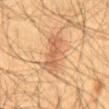| field | value |
|---|---|
| biopsy status | catalogued during a skin exam; not biopsied |
| image-analysis metrics | a lesion area of about 14 mm² and two-axis asymmetry of about 0.3; a lesion color around L≈57 a*≈20 b*≈35 in CIELAB, roughly 9 lightness units darker than nearby skin, and a normalized lesion–skin contrast near 6 |
| lighting | cross-polarized illumination |
| patient | male, about 60 years old |
| site | the abdomen |
| diameter | ~6 mm (longest diameter) |
| acquisition | total-body-photography crop, ~15 mm field of view |